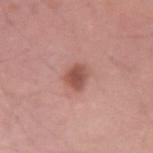{"biopsy_status": "not biopsied; imaged during a skin examination", "site": "arm", "lighting": "white-light", "image": {"source": "total-body photography crop", "field_of_view_mm": 15}, "patient": {"sex": "male", "age_approx": 35}}